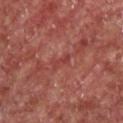Q: Was a biopsy performed?
A: imaged on a skin check; not biopsied
Q: What lighting was used for the tile?
A: cross-polarized illumination
Q: What is the imaging modality?
A: total-body-photography crop, ~15 mm field of view
Q: Patient demographics?
A: male, roughly 55 years of age
Q: Lesion location?
A: the chest
Q: How large is the lesion?
A: ~2.5 mm (longest diameter)
Q: Automated lesion metrics?
A: an eccentricity of roughly 0.9 and a shape-asymmetry score of about 0.4 (0 = symmetric); a mean CIELAB color near L≈36 a*≈28 b*≈23, a lesion–skin lightness drop of about 5, and a lesion-to-skin contrast of about 5 (normalized; higher = more distinct); a border-irregularity rating of about 5/10, internal color variation of about 0 on a 0–10 scale, and a peripheral color-asymmetry measure near 0; a lesion-detection confidence of about 65/100